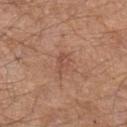This lesion was catalogued during total-body skin photography and was not selected for biopsy.
Automated image analysis of the tile measured a shape eccentricity near 0.85 and two-axis asymmetry of about 0.35. And it measured about 7 CIELAB-L* units darker than the surrounding skin and a lesion-to-skin contrast of about 5 (normalized; higher = more distinct).
The lesion's longest dimension is about 3 mm.
A close-up tile cropped from a whole-body skin photograph, about 15 mm across.
This is a white-light tile.
The patient is a male aged around 65.
The lesion is on the left upper arm.